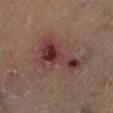diameter=about 6.5 mm | tile lighting=cross-polarized illumination | anatomic site=the right lower leg | image source=15 mm crop, total-body photography | patient=male, about 70 years old | automated metrics=border irregularity of about 7.5 on a 0–10 scale, a within-lesion color-variation index near 10/10, and a peripheral color-asymmetry measure near 4; a detector confidence of about 100 out of 100 that the crop contains a lesion.